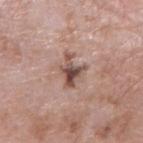The lesion was tiled from a total-body skin photograph and was not biopsied. A male patient, aged around 75. Automated tile analysis of the lesion measured an eccentricity of roughly 0.75 and a symmetry-axis asymmetry near 0.55. And it measured a mean CIELAB color near L≈50 a*≈19 b*≈23, about 13 CIELAB-L* units darker than the surrounding skin, and a lesion-to-skin contrast of about 9.5 (normalized; higher = more distinct). The software also gave a lesion-detection confidence of about 100/100. A region of skin cropped from a whole-body photographic capture, roughly 15 mm wide. On the right forearm. The lesion's longest dimension is about 4 mm.